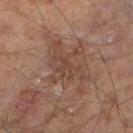Clinical impression: Captured during whole-body skin photography for melanoma surveillance; the lesion was not biopsied. Context: This image is a 15 mm lesion crop taken from a total-body photograph. A male patient, aged approximately 65. About 4 mm across. The lesion is located on the leg. Automated tile analysis of the lesion measured border irregularity of about 8 on a 0–10 scale, a within-lesion color-variation index near 2.5/10, and a peripheral color-asymmetry measure near 1.